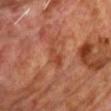workup = total-body-photography surveillance lesion; no biopsy | image-analysis metrics = a classifier nevus-likeness of about 0/100 and lesion-presence confidence of about 90/100 | image = total-body-photography crop, ~15 mm field of view | patient = male, roughly 70 years of age | lighting = cross-polarized | size = ≈4.5 mm | body site = the chest.